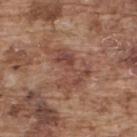Assessment: No biopsy was performed on this lesion — it was imaged during a full skin examination and was not determined to be concerning. Background: Cropped from a total-body skin-imaging series; the visible field is about 15 mm. A male patient, aged 73–77. From the back.